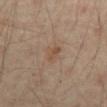No biopsy was performed on this lesion — it was imaged during a full skin examination and was not determined to be concerning.
A male subject, roughly 45 years of age.
Imaged with cross-polarized lighting.
From the abdomen.
Automated image analysis of the tile measured a lesion area of about 4 mm² and a shape eccentricity near 0.75. The analysis additionally found radial color variation of about 0.5. It also reported a nevus-likeness score of about 0/100.
A roughly 15 mm field-of-view crop from a total-body skin photograph.
Measured at roughly 2.5 mm in maximum diameter.